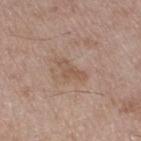A region of skin cropped from a whole-body photographic capture, roughly 15 mm wide. A male subject, in their 50s. The lesion is on the right thigh.